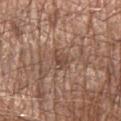From the left forearm.
Cropped from a whole-body photographic skin survey; the tile spans about 15 mm.
An algorithmic analysis of the crop reported a footprint of about 3.5 mm². The analysis additionally found a lesion color around L≈47 a*≈18 b*≈27 in CIELAB and a normalized lesion–skin contrast near 6. The analysis additionally found a border-irregularity index near 3.5/10, a color-variation rating of about 2/10, and radial color variation of about 0.5. The analysis additionally found a lesion-detection confidence of about 80/100.
A male subject aged approximately 70.
About 2.5 mm across.